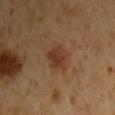The lesion was photographed on a routine skin check and not biopsied; there is no pathology result. A close-up tile cropped from a whole-body skin photograph, about 15 mm across. Located on the right upper arm. About 4 mm across. The lesion-visualizer software estimated an area of roughly 7 mm² and a symmetry-axis asymmetry near 0.25. It also reported a lesion–skin lightness drop of about 8 and a normalized border contrast of about 7.5. The patient is a male aged 48 to 52. The tile uses cross-polarized illumination.